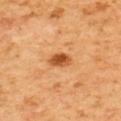No biopsy was performed on this lesion — it was imaged during a full skin examination and was not determined to be concerning.
A lesion tile, about 15 mm wide, cut from a 3D total-body photograph.
On the back.
Captured under cross-polarized illumination.
Longest diameter approximately 2.5 mm.
A male patient, aged approximately 60.